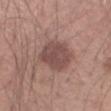- biopsy status — total-body-photography surveillance lesion; no biopsy
- image source — total-body-photography crop, ~15 mm field of view
- tile lighting — white-light
- location — the left forearm
- size — ~4 mm (longest diameter)
- automated metrics — an outline eccentricity of about 0.55 (0 = round, 1 = elongated) and a shape-asymmetry score of about 0.15 (0 = symmetric); a lesion color around L≈48 a*≈19 b*≈21 in CIELAB, a lesion–skin lightness drop of about 10, and a lesion-to-skin contrast of about 8 (normalized; higher = more distinct); a border-irregularity rating of about 1.5/10, a color-variation rating of about 2.5/10, and peripheral color asymmetry of about 1; a nevus-likeness score of about 20/100 and a detector confidence of about 100 out of 100 that the crop contains a lesion
- patient — male, approximately 45 years of age